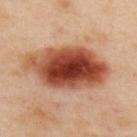Q: Was this lesion biopsied?
A: catalogued during a skin exam; not biopsied
Q: Lesion location?
A: the upper back
Q: Automated lesion metrics?
A: a lesion area of about 36 mm², an outline eccentricity of about 0.85 (0 = round, 1 = elongated), and a symmetry-axis asymmetry near 0.15; a border-irregularity index near 2/10, a color-variation rating of about 10/10, and radial color variation of about 3.5; an automated nevus-likeness rating near 100 out of 100 and a lesion-detection confidence of about 100/100
Q: Lesion size?
A: ≈9.5 mm
Q: Who is the patient?
A: female, aged around 40
Q: How was this image acquired?
A: total-body-photography crop, ~15 mm field of view